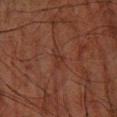Image and clinical context:
The lesion is located on the left forearm. The subject is a male aged around 65. A 15 mm close-up tile from a total-body photography series done for melanoma screening. About 2.5 mm across. Captured under cross-polarized illumination.